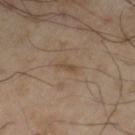follow-up: no biopsy performed (imaged during a skin exam) | TBP lesion metrics: a border-irregularity rating of about 3.5/10 and radial color variation of about 0; a nevus-likeness score of about 0/100 and a detector confidence of about 100 out of 100 that the crop contains a lesion | subject: male, approximately 55 years of age | size: about 2.5 mm | acquisition: 15 mm crop, total-body photography | anatomic site: the left thigh.